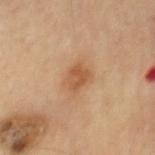{
  "biopsy_status": "not biopsied; imaged during a skin examination",
  "patient": {
    "sex": "male",
    "age_approx": "85+"
  },
  "lighting": "cross-polarized",
  "image": {
    "source": "total-body photography crop",
    "field_of_view_mm": 15
  },
  "lesion_size": {
    "long_diameter_mm_approx": 3.0
  },
  "site": "chest"
}